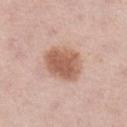Q: Is there a histopathology result?
A: no biopsy performed (imaged during a skin exam)
Q: What is the imaging modality?
A: ~15 mm crop, total-body skin-cancer survey
Q: Patient demographics?
A: female, in their mid- to late 60s
Q: Lesion location?
A: the left lower leg